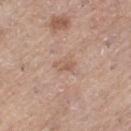Imaged during a routine full-body skin examination; the lesion was not biopsied and no histopathology is available.
Automated tile analysis of the lesion measured a footprint of about 3.5 mm². The analysis additionally found a border-irregularity rating of about 2.5/10 and a color-variation rating of about 3/10. The analysis additionally found an automated nevus-likeness rating near 0 out of 100 and a detector confidence of about 100 out of 100 that the crop contains a lesion.
The subject is a female aged 63 to 67.
A 15 mm crop from a total-body photograph taken for skin-cancer surveillance.
The tile uses white-light illumination.
The lesion is on the left thigh.